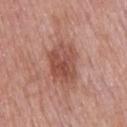follow-up: catalogued during a skin exam; not biopsied
anatomic site: the head or neck
image source: ~15 mm crop, total-body skin-cancer survey
automated metrics: a lesion area of about 14 mm², an eccentricity of roughly 0.65, and a symmetry-axis asymmetry near 0.2; an automated nevus-likeness rating near 5 out of 100 and lesion-presence confidence of about 100/100
lesion size: ~4.5 mm (longest diameter)
patient: male, aged 53–57